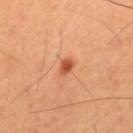Recorded during total-body skin imaging; not selected for excision or biopsy.
A region of skin cropped from a whole-body photographic capture, roughly 15 mm wide.
On the mid back.
Automated image analysis of the tile measured a classifier nevus-likeness of about 95/100.
A male patient aged 63 to 67.